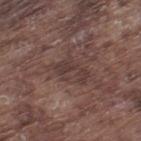<lesion>
<biopsy_status>not biopsied; imaged during a skin examination</biopsy_status>
<lesion_size>
  <long_diameter_mm_approx>4.0</long_diameter_mm_approx>
</lesion_size>
<image>
  <source>total-body photography crop</source>
  <field_of_view_mm>15</field_of_view_mm>
</image>
<lighting>white-light</lighting>
<automated_metrics>
  <cielab_L>37</cielab_L>
  <cielab_a>17</cielab_a>
  <cielab_b>19</cielab_b>
  <vs_skin_darker_L>7.0</vs_skin_darker_L>
  <vs_skin_contrast_norm>6.0</vs_skin_contrast_norm>
  <nevus_likeness_0_100>0</nevus_likeness_0_100>
  <lesion_detection_confidence_0_100>65</lesion_detection_confidence_0_100>
</automated_metrics>
<site>left thigh</site>
<patient>
  <sex>male</sex>
  <age_approx>75</age_approx>
</patient>
</lesion>